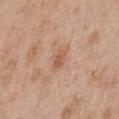This lesion was catalogued during total-body skin photography and was not selected for biopsy. This is a white-light tile. An algorithmic analysis of the crop reported border irregularity of about 2.5 on a 0–10 scale, a color-variation rating of about 1.5/10, and a peripheral color-asymmetry measure near 0.5. The analysis additionally found an automated nevus-likeness rating near 0 out of 100 and lesion-presence confidence of about 100/100. Measured at roughly 2.5 mm in maximum diameter. A 15 mm crop from a total-body photograph taken for skin-cancer surveillance. A male subject, roughly 65 years of age. The lesion is on the front of the torso.